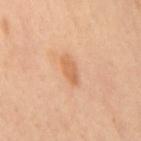| key | value |
|---|---|
| workup | catalogued during a skin exam; not biopsied |